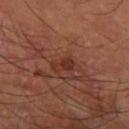notes = no biopsy performed (imaged during a skin exam); subject = male, aged around 65; body site = the right forearm; illumination = cross-polarized; image = 15 mm crop, total-body photography; size = ~2.5 mm (longest diameter).